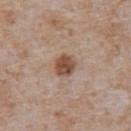- automated metrics — about 13 CIELAB-L* units darker than the surrounding skin and a normalized lesion–skin contrast near 9.5; a border-irregularity rating of about 1/10, a within-lesion color-variation index near 2.5/10, and radial color variation of about 1; a classifier nevus-likeness of about 95/100
- acquisition — ~15 mm crop, total-body skin-cancer survey
- size — ~2.5 mm (longest diameter)
- patient — male, aged 63 to 67
- body site — the front of the torso
- lighting — white-light illumination